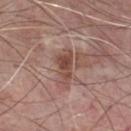Clinical impression:
No biopsy was performed on this lesion — it was imaged during a full skin examination and was not determined to be concerning.
Context:
A male patient, aged approximately 75. A close-up tile cropped from a whole-body skin photograph, about 15 mm across. On the front of the torso. An algorithmic analysis of the crop reported a lesion area of about 6 mm², a shape eccentricity near 0.9, and a symmetry-axis asymmetry near 0.45.